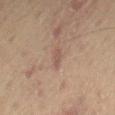Clinical impression:
No biopsy was performed on this lesion — it was imaged during a full skin examination and was not determined to be concerning.
Background:
The total-body-photography lesion software estimated a mean CIELAB color near L≈47 a*≈17 b*≈22 and roughly 7 lightness units darker than nearby skin. This is a cross-polarized tile. A male subject, aged around 65. A roughly 15 mm field-of-view crop from a total-body skin photograph. About 2.5 mm across. On the right thigh.